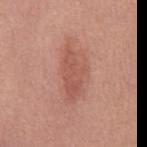Clinical impression: No biopsy was performed on this lesion — it was imaged during a full skin examination and was not determined to be concerning. Image and clinical context: The tile uses white-light illumination. Automated image analysis of the tile measured a border-irregularity index near 4/10 and radial color variation of about 1. Longest diameter approximately 6 mm. The subject is a female aged 48 to 52. A close-up tile cropped from a whole-body skin photograph, about 15 mm across. From the chest.